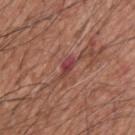{"biopsy_status": "not biopsied; imaged during a skin examination", "image": {"source": "total-body photography crop", "field_of_view_mm": 15}, "patient": {"sex": "male", "age_approx": 60}, "site": "upper back"}